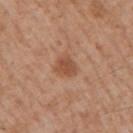Notes:
– notes: imaged on a skin check; not biopsied
– site: the right upper arm
– image-analysis metrics: a within-lesion color-variation index near 2/10
– size: about 2.5 mm
– patient: male, aged approximately 60
– tile lighting: white-light illumination
– image: ~15 mm crop, total-body skin-cancer survey